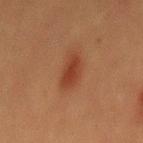Imaged during a routine full-body skin examination; the lesion was not biopsied and no histopathology is available. The lesion's longest dimension is about 4 mm. The lesion is located on the mid back. A male subject, aged 38–42. A close-up tile cropped from a whole-body skin photograph, about 15 mm across.